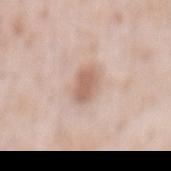biopsy status: total-body-photography surveillance lesion; no biopsy | patient: male, aged around 50 | illumination: white-light | lesion diameter: ~3.5 mm (longest diameter) | image-analysis metrics: a lesion area of about 6 mm², an outline eccentricity of about 0.85 (0 = round, 1 = elongated), and two-axis asymmetry of about 0.15 | body site: the mid back | image source: ~15 mm crop, total-body skin-cancer survey.